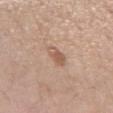Q: Is there a histopathology result?
A: total-body-photography surveillance lesion; no biopsy
Q: What are the patient's age and sex?
A: female, approximately 40 years of age
Q: Automated lesion metrics?
A: a mean CIELAB color near L≈57 a*≈20 b*≈28, a lesion–skin lightness drop of about 9, and a normalized border contrast of about 6.5
Q: Where on the body is the lesion?
A: the arm
Q: How was the tile lit?
A: white-light illumination
Q: What is the lesion's diameter?
A: about 2.5 mm
Q: What is the imaging modality?
A: total-body-photography crop, ~15 mm field of view